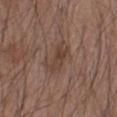biopsy status: catalogued during a skin exam; not biopsied | location: the right forearm | subject: male, in their mid- to late 40s | lesion size: ~4.5 mm (longest diameter) | imaging modality: 15 mm crop, total-body photography.